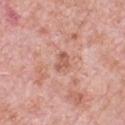Assessment: No biopsy was performed on this lesion — it was imaged during a full skin examination and was not determined to be concerning. Clinical summary: The lesion's longest dimension is about 3 mm. From the front of the torso. A male patient, aged approximately 80. The lesion-visualizer software estimated an outline eccentricity of about 0.8 (0 = round, 1 = elongated) and a shape-asymmetry score of about 0.25 (0 = symmetric). The analysis additionally found an average lesion color of about L≈58 a*≈24 b*≈29 (CIELAB) and a lesion-to-skin contrast of about 6 (normalized; higher = more distinct). It also reported a within-lesion color-variation index near 1.5/10. A 15 mm close-up tile from a total-body photography series done for melanoma screening. The tile uses white-light illumination.